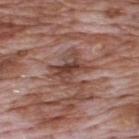Context: Longest diameter approximately 3.5 mm. The tile uses white-light illumination. The lesion is on the upper back. A male subject about 70 years old. Cropped from a total-body skin-imaging series; the visible field is about 15 mm.